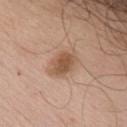Assessment:
Imaged during a routine full-body skin examination; the lesion was not biopsied and no histopathology is available.
Image and clinical context:
The patient is a male aged around 60. Located on the arm. Captured under white-light illumination. Cropped from a total-body skin-imaging series; the visible field is about 15 mm. About 4 mm across.